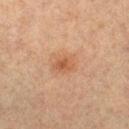Impression: The lesion was tiled from a total-body skin photograph and was not biopsied. Background: A 15 mm crop from a total-body photograph taken for skin-cancer surveillance. Automated tile analysis of the lesion measured a shape eccentricity near 0.75. The analysis additionally found a lesion color around L≈50 a*≈21 b*≈33 in CIELAB, a lesion–skin lightness drop of about 7, and a lesion-to-skin contrast of about 6.5 (normalized; higher = more distinct). The lesion's longest dimension is about 3 mm. A female patient, aged 58–62. The lesion is located on the right lower leg. This is a cross-polarized tile.